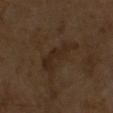- notes: catalogued during a skin exam; not biopsied
- diameter: about 5.5 mm
- image source: ~15 mm tile from a whole-body skin photo
- tile lighting: cross-polarized
- patient: male, about 65 years old
- TBP lesion metrics: a lesion color around L≈23 a*≈13 b*≈23 in CIELAB, roughly 5 lightness units darker than nearby skin, and a normalized border contrast of about 6.5; border irregularity of about 7 on a 0–10 scale and a peripheral color-asymmetry measure near 0.5; a nevus-likeness score of about 0/100 and lesion-presence confidence of about 65/100